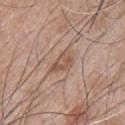Assessment:
Part of a total-body skin-imaging series; this lesion was reviewed on a skin check and was not flagged for biopsy.
Image and clinical context:
An algorithmic analysis of the crop reported an eccentricity of roughly 0.8 and a shape-asymmetry score of about 0.3 (0 = symmetric). A 15 mm close-up extracted from a 3D total-body photography capture. Measured at roughly 3.5 mm in maximum diameter. Located on the chest. A male subject roughly 50 years of age. Captured under white-light illumination.